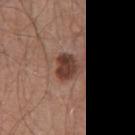Notes:
- biopsy status — no biopsy performed (imaged during a skin exam)
- site — the abdomen
- imaging modality — 15 mm crop, total-body photography
- subject — male, roughly 65 years of age
- TBP lesion metrics — a mean CIELAB color near L≈40 a*≈20 b*≈24, about 13 CIELAB-L* units darker than the surrounding skin, and a normalized border contrast of about 10.5; border irregularity of about 2.5 on a 0–10 scale, internal color variation of about 4 on a 0–10 scale, and radial color variation of about 1.5; an automated nevus-likeness rating near 30 out of 100 and a detector confidence of about 100 out of 100 that the crop contains a lesion